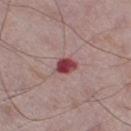location — the left thigh | patient — male, in their mid-70s | image source — total-body-photography crop, ~15 mm field of view | lesion size — about 2.5 mm.